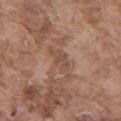Assessment:
The lesion was tiled from a total-body skin photograph and was not biopsied.
Acquisition and patient details:
Imaged with white-light lighting. A 15 mm close-up tile from a total-body photography series done for melanoma screening. The lesion-visualizer software estimated an area of roughly 4.5 mm² and an eccentricity of roughly 0.85. It also reported an average lesion color of about L≈49 a*≈19 b*≈27 (CIELAB), a lesion–skin lightness drop of about 7, and a normalized lesion–skin contrast near 5.5. The analysis additionally found a nevus-likeness score of about 0/100. A male patient aged approximately 75. From the mid back.